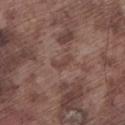notes=imaged on a skin check; not biopsied | tile lighting=white-light illumination | automated lesion analysis=a lesion color around L≈42 a*≈18 b*≈23 in CIELAB; an automated nevus-likeness rating near 0 out of 100 | imaging modality=~15 mm tile from a whole-body skin photo | lesion size=≈3 mm | patient=male, aged approximately 75 | location=the leg.